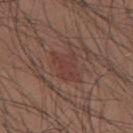Part of a total-body skin-imaging series; this lesion was reviewed on a skin check and was not flagged for biopsy.